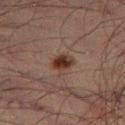– workup · no biopsy performed (imaged during a skin exam)
– subject · male, in their 50s
– anatomic site · the left thigh
– lighting · cross-polarized illumination
– imaging modality · ~15 mm crop, total-body skin-cancer survey
– diameter · ≈2.5 mm